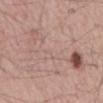Captured during whole-body skin photography for melanoma surveillance; the lesion was not biopsied.